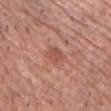Acquisition and patient details:
Located on the chest. A 15 mm close-up tile from a total-body photography series done for melanoma screening. A male patient aged 78 to 82.